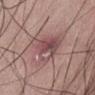anatomic site: the abdomen
automated lesion analysis: an area of roughly 16 mm², an eccentricity of roughly 0.7, and a shape-asymmetry score of about 0.45 (0 = symmetric); a mean CIELAB color near L≈50 a*≈21 b*≈18, a lesion–skin lightness drop of about 9, and a normalized lesion–skin contrast near 7; border irregularity of about 5.5 on a 0–10 scale, internal color variation of about 7.5 on a 0–10 scale, and radial color variation of about 2.5; a nevus-likeness score of about 0/100 and a lesion-detection confidence of about 100/100
acquisition: total-body-photography crop, ~15 mm field of view
lesion size: about 6 mm
lighting: white-light illumination
subject: male, aged approximately 55